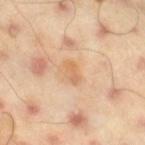biopsy status — no biopsy performed (imaged during a skin exam); imaging modality — ~15 mm tile from a whole-body skin photo; subject — male, roughly 45 years of age; site — the right thigh; lesion diameter — ~2.5 mm (longest diameter); tile lighting — cross-polarized illumination.